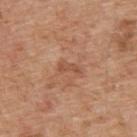follow-up: total-body-photography surveillance lesion; no biopsy | subject: male, roughly 65 years of age | diameter: about 2.5 mm | image-analysis metrics: a footprint of about 2 mm², an eccentricity of roughly 0.95, and a shape-asymmetry score of about 0.4 (0 = symmetric); internal color variation of about 0 on a 0–10 scale and radial color variation of about 0; a classifier nevus-likeness of about 0/100 and lesion-presence confidence of about 100/100 | site: the upper back | image source: 15 mm crop, total-body photography.